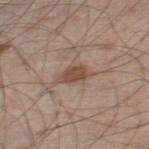Imaged during a routine full-body skin examination; the lesion was not biopsied and no histopathology is available. A male patient, aged 58–62. The tile uses white-light illumination. Approximately 3.5 mm at its widest. A lesion tile, about 15 mm wide, cut from a 3D total-body photograph. An algorithmic analysis of the crop reported a lesion area of about 5.5 mm², an eccentricity of roughly 0.8, and a symmetry-axis asymmetry near 0.3. And it measured a mean CIELAB color near L≈48 a*≈18 b*≈26, a lesion–skin lightness drop of about 10, and a lesion-to-skin contrast of about 8 (normalized; higher = more distinct). It also reported border irregularity of about 3.5 on a 0–10 scale, a within-lesion color-variation index near 2/10, and a peripheral color-asymmetry measure near 1. On the right thigh.